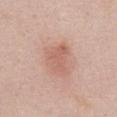illumination = white-light | subject = female, aged approximately 50 | automated metrics = a lesion color around L≈60 a*≈23 b*≈27 in CIELAB, roughly 8 lightness units darker than nearby skin, and a normalized lesion–skin contrast near 5.5; an automated nevus-likeness rating near 25 out of 100 and a lesion-detection confidence of about 100/100 | body site = the abdomen | image = 15 mm crop, total-body photography.